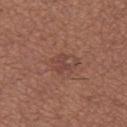{"biopsy_status": "not biopsied; imaged during a skin examination", "site": "left thigh", "automated_metrics": {"cielab_L": 43, "cielab_a": 22, "cielab_b": 25, "vs_skin_darker_L": 6.0, "vs_skin_contrast_norm": 5.5, "nevus_likeness_0_100": 0}, "patient": {"sex": "female", "age_approx": 25}, "image": {"source": "total-body photography crop", "field_of_view_mm": 15}, "lesion_size": {"long_diameter_mm_approx": 3.0}, "lighting": "white-light"}